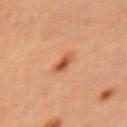{
  "biopsy_status": "not biopsied; imaged during a skin examination",
  "image": {
    "source": "total-body photography crop",
    "field_of_view_mm": 15
  },
  "site": "left upper arm",
  "automated_metrics": {
    "vs_skin_darker_L": 12.0,
    "vs_skin_contrast_norm": 8.5
  },
  "patient": {
    "sex": "female",
    "age_approx": 35
  },
  "lesion_size": {
    "long_diameter_mm_approx": 2.5
  }
}